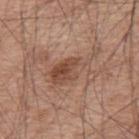No biopsy was performed on this lesion — it was imaged during a full skin examination and was not determined to be concerning.
The patient is a male in their mid-50s.
Located on the upper back.
Measured at roughly 6.5 mm in maximum diameter.
A lesion tile, about 15 mm wide, cut from a 3D total-body photograph.
The tile uses white-light illumination.